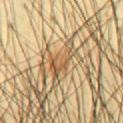workup: total-body-photography surveillance lesion; no biopsy
location: the abdomen
image: 15 mm crop, total-body photography
illumination: cross-polarized
patient: male, aged 43–47